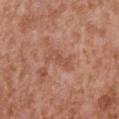{
  "biopsy_status": "not biopsied; imaged during a skin examination",
  "patient": {
    "sex": "male",
    "age_approx": 45
  },
  "site": "chest",
  "image": {
    "source": "total-body photography crop",
    "field_of_view_mm": 15
  }
}